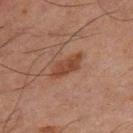biopsy_status: not biopsied; imaged during a skin examination
site: right thigh
patient:
  sex: male
  age_approx: 65
lighting: cross-polarized
image:
  source: total-body photography crop
  field_of_view_mm: 15
automated_metrics:
  area_mm2_approx: 7.0
  eccentricity: 0.9
  shape_asymmetry: 0.3
  nevus_likeness_0_100: 30
  lesion_detection_confidence_0_100: 100
lesion_size:
  long_diameter_mm_approx: 4.5